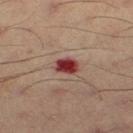Q: Is there a histopathology result?
A: total-body-photography surveillance lesion; no biopsy
Q: Lesion size?
A: about 2.5 mm
Q: What is the anatomic site?
A: the left thigh
Q: Automated lesion metrics?
A: a shape eccentricity near 0.65 and a symmetry-axis asymmetry near 0.2
Q: What is the imaging modality?
A: ~15 mm tile from a whole-body skin photo
Q: Patient demographics?
A: male, about 55 years old
Q: How was the tile lit?
A: cross-polarized